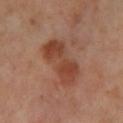Imaged during a routine full-body skin examination; the lesion was not biopsied and no histopathology is available. This is a cross-polarized tile. About 6 mm across. A 15 mm crop from a total-body photograph taken for skin-cancer surveillance. The lesion is located on the leg. The patient is a female aged around 55.